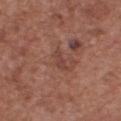biopsy status = imaged on a skin check; not biopsied.